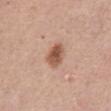Q: Illumination type?
A: white-light
Q: Automated lesion metrics?
A: a lesion area of about 6 mm², a shape eccentricity near 0.75, and a symmetry-axis asymmetry near 0.2; an average lesion color of about L≈55 a*≈22 b*≈31 (CIELAB), roughly 13 lightness units darker than nearby skin, and a normalized lesion–skin contrast near 9; a border-irregularity rating of about 2/10, a color-variation rating of about 4.5/10, and peripheral color asymmetry of about 1.5; a nevus-likeness score of about 95/100 and a detector confidence of about 100 out of 100 that the crop contains a lesion
Q: What kind of image is this?
A: total-body-photography crop, ~15 mm field of view
Q: Lesion location?
A: the front of the torso
Q: How large is the lesion?
A: ≈3.5 mm
Q: What are the patient's age and sex?
A: female, aged 58–62